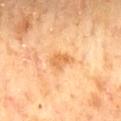No biopsy was performed on this lesion — it was imaged during a full skin examination and was not determined to be concerning.